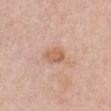Findings:
• follow-up · catalogued during a skin exam; not biopsied
• illumination · white-light
• diameter · about 3 mm
• imaging modality · total-body-photography crop, ~15 mm field of view
• anatomic site · the chest
• subject · female, about 50 years old
• automated lesion analysis · an automated nevus-likeness rating near 5 out of 100 and a detector confidence of about 100 out of 100 that the crop contains a lesion